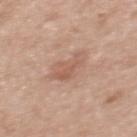Imaged with white-light lighting.
About 4 mm across.
A female subject aged 48–52.
A region of skin cropped from a whole-body photographic capture, roughly 15 mm wide.
From the upper back.